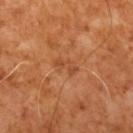<lesion>
  <lesion_size>
    <long_diameter_mm_approx>3.0</long_diameter_mm_approx>
  </lesion_size>
  <image>
    <source>total-body photography crop</source>
    <field_of_view_mm>15</field_of_view_mm>
  </image>
  <automated_metrics>
    <border_irregularity_0_10>3.5</border_irregularity_0_10>
    <peripheral_color_asymmetry>0.0</peripheral_color_asymmetry>
  </automated_metrics>
  <lighting>cross-polarized</lighting>
  <patient>
    <sex>male</sex>
    <age_approx>65</age_approx>
  </patient>
</lesion>